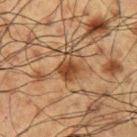This lesion was catalogued during total-body skin photography and was not selected for biopsy.
This image is a 15 mm lesion crop taken from a total-body photograph.
Located on the left upper arm.
Longest diameter approximately 3 mm.
Imaged with cross-polarized lighting.
The patient is a male about 60 years old.